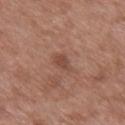| feature | finding |
|---|---|
| notes | no biopsy performed (imaged during a skin exam) |
| image source | ~15 mm crop, total-body skin-cancer survey |
| anatomic site | the mid back |
| subject | male, roughly 50 years of age |